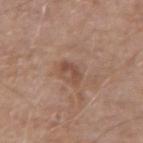follow-up = no biopsy performed (imaged during a skin exam)
illumination = white-light
acquisition = ~15 mm crop, total-body skin-cancer survey
anatomic site = the left forearm
patient = male, aged around 70
lesion size = ≈3 mm
automated metrics = a mean CIELAB color near L≈48 a*≈20 b*≈28; a border-irregularity rating of about 4.5/10, internal color variation of about 0.5 on a 0–10 scale, and peripheral color asymmetry of about 0; a nevus-likeness score of about 0/100 and lesion-presence confidence of about 100/100